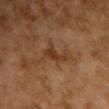{
  "biopsy_status": "not biopsied; imaged during a skin examination",
  "lesion_size": {
    "long_diameter_mm_approx": 3.5
  },
  "site": "front of the torso",
  "image": {
    "source": "total-body photography crop",
    "field_of_view_mm": 15
  },
  "patient": {
    "sex": "female",
    "age_approx": 60
  }
}